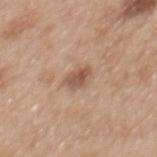Q: Was a biopsy performed?
A: imaged on a skin check; not biopsied
Q: What is the imaging modality?
A: ~15 mm crop, total-body skin-cancer survey
Q: Who is the patient?
A: female, aged around 40
Q: What is the anatomic site?
A: the mid back